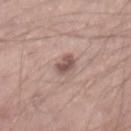| field | value |
|---|---|
| workup | no biopsy performed (imaged during a skin exam) |
| diameter | about 2.5 mm |
| image source | 15 mm crop, total-body photography |
| image-analysis metrics | an average lesion color of about L≈53 a*≈18 b*≈21 (CIELAB), a lesion–skin lightness drop of about 12, and a lesion-to-skin contrast of about 8.5 (normalized; higher = more distinct); border irregularity of about 2 on a 0–10 scale and a color-variation rating of about 2.5/10 |
| subject | male, in their mid-40s |
| body site | the left forearm |